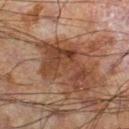The lesion was photographed on a routine skin check and not biopsied; there is no pathology result. Automated tile analysis of the lesion measured an area of roughly 18 mm² and two-axis asymmetry of about 0.55. It also reported a border-irregularity rating of about 8.5/10 and peripheral color asymmetry of about 1. Captured under cross-polarized illumination. Approximately 7.5 mm at its widest. A male patient aged around 60. A lesion tile, about 15 mm wide, cut from a 3D total-body photograph. Located on the left leg.